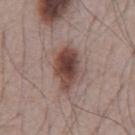{
  "biopsy_status": "not biopsied; imaged during a skin examination",
  "patient": {
    "sex": "male",
    "age_approx": 65
  },
  "lighting": "white-light",
  "site": "chest",
  "image": {
    "source": "total-body photography crop",
    "field_of_view_mm": 15
  },
  "lesion_size": {
    "long_diameter_mm_approx": 5.0
  }
}